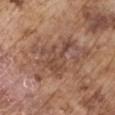diameter = ~5 mm (longest diameter); subject = male, aged 73–77; illumination = white-light illumination; image = 15 mm crop, total-body photography; location = the right upper arm.